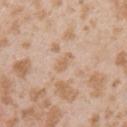biopsy status: no biopsy performed (imaged during a skin exam) | site: the left upper arm | lesion diameter: about 2.5 mm | subject: female, about 25 years old | image: total-body-photography crop, ~15 mm field of view.